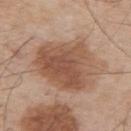Captured during whole-body skin photography for melanoma surveillance; the lesion was not biopsied.
This is a white-light tile.
The patient is a male about 55 years old.
A 15 mm crop from a total-body photograph taken for skin-cancer surveillance.
The total-body-photography lesion software estimated a lesion area of about 31 mm², a shape eccentricity near 0.65, and a symmetry-axis asymmetry near 0.2. The software also gave a border-irregularity index near 3/10, a within-lesion color-variation index near 4.5/10, and peripheral color asymmetry of about 1.5.
From the upper back.
The recorded lesion diameter is about 7.5 mm.